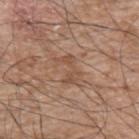Case summary:
– patient: male, roughly 65 years of age
– tile lighting: white-light
– imaging modality: ~15 mm tile from a whole-body skin photo
– anatomic site: the upper back
– size: ~3.5 mm (longest diameter)
– image-analysis metrics: a lesion area of about 5 mm², an outline eccentricity of about 0.8 (0 = round, 1 = elongated), and two-axis asymmetry of about 0.45; a border-irregularity rating of about 7.5/10 and a within-lesion color-variation index near 2/10